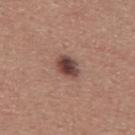illumination = white-light illumination
imaging modality = 15 mm crop, total-body photography
site = the mid back
subject = male, about 45 years old